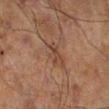Q: Is there a histopathology result?
A: imaged on a skin check; not biopsied
Q: Patient demographics?
A: male, approximately 70 years of age
Q: What did automated image analysis measure?
A: a lesion area of about 3.5 mm², an eccentricity of roughly 0.8, and a shape-asymmetry score of about 0.4 (0 = symmetric)
Q: Lesion location?
A: the right lower leg
Q: What is the imaging modality?
A: total-body-photography crop, ~15 mm field of view
Q: How large is the lesion?
A: about 2.5 mm
Q: How was the tile lit?
A: cross-polarized illumination